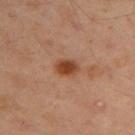Case summary:
– workup · catalogued during a skin exam; not biopsied
– TBP lesion metrics · a lesion color around L≈43 a*≈25 b*≈34 in CIELAB and roughly 12 lightness units darker than nearby skin; a border-irregularity index near 1.5/10, a within-lesion color-variation index near 3/10, and peripheral color asymmetry of about 1; a lesion-detection confidence of about 100/100
– location · the left arm
– image · ~15 mm tile from a whole-body skin photo
– lesion size · ≈3 mm
– subject · male, aged approximately 50
– illumination · cross-polarized illumination